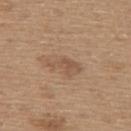workup — imaged on a skin check; not biopsied
patient — male, approximately 65 years of age
body site — the upper back
size — ≈4 mm
image — ~15 mm crop, total-body skin-cancer survey